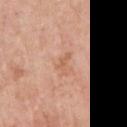- imaging modality — ~15 mm crop, total-body skin-cancer survey
- TBP lesion metrics — a border-irregularity index near 6.5/10, internal color variation of about 0 on a 0–10 scale, and radial color variation of about 0
- subject — male, roughly 60 years of age
- tile lighting — white-light
- lesion diameter — ~2.5 mm (longest diameter)
- site — the left upper arm